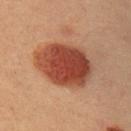Notes:
– site: the right upper arm
– subject: male, aged around 40
– acquisition: total-body-photography crop, ~15 mm field of view